A female patient aged 38 to 42, on the mid back, a 15 mm close-up tile from a total-body photography series done for melanoma screening — 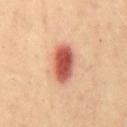Conclusion:
The biopsy diagnosis was an atypical melanocytic neoplasm, classified as a borderline lesion of uncertain malignant potential.